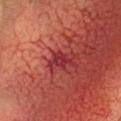Assessment:
Captured during whole-body skin photography for melanoma surveillance; the lesion was not biopsied.
Acquisition and patient details:
Approximately 2.5 mm at its widest. The patient is a male approximately 35 years of age. The lesion is on the head or neck. This image is a 15 mm lesion crop taken from a total-body photograph. The lesion-visualizer software estimated an area of roughly 4 mm². The software also gave an average lesion color of about L≈27 a*≈32 b*≈19 (CIELAB) and a normalized lesion–skin contrast near 8.5. It also reported a classifier nevus-likeness of about 0/100 and lesion-presence confidence of about 90/100.